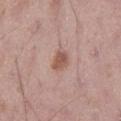Imaged during a routine full-body skin examination; the lesion was not biopsied and no histopathology is available.
Automated image analysis of the tile measured a lesion area of about 5 mm², an outline eccentricity of about 0.7 (0 = round, 1 = elongated), and two-axis asymmetry of about 0.3. The software also gave a lesion color around L≈55 a*≈20 b*≈26 in CIELAB, a lesion–skin lightness drop of about 10, and a lesion-to-skin contrast of about 7.5 (normalized; higher = more distinct). The analysis additionally found a color-variation rating of about 2/10 and radial color variation of about 1. It also reported a lesion-detection confidence of about 100/100.
Located on the left thigh.
Captured under white-light illumination.
A male patient, in their 50s.
A 15 mm close-up extracted from a 3D total-body photography capture.
Longest diameter approximately 3 mm.